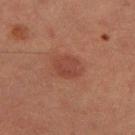Clinical impression:
This lesion was catalogued during total-body skin photography and was not selected for biopsy.
Acquisition and patient details:
An algorithmic analysis of the crop reported a lesion color around L≈35 a*≈22 b*≈24 in CIELAB, roughly 6 lightness units darker than nearby skin, and a normalized lesion–skin contrast near 6. On the left thigh. A 15 mm close-up extracted from a 3D total-body photography capture. A female patient, aged 38 to 42. This is a cross-polarized tile. The recorded lesion diameter is about 3.5 mm.